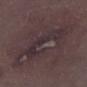biopsy_status: not biopsied; imaged during a skin examination